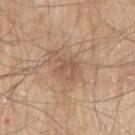notes = imaged on a skin check; not biopsied
subject = male, aged around 80
image = 15 mm crop, total-body photography
tile lighting = white-light illumination
location = the right upper arm
automated lesion analysis = a peripheral color-asymmetry measure near 0